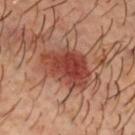No biopsy was performed on this lesion — it was imaged during a full skin examination and was not determined to be concerning.
Captured under cross-polarized illumination.
The lesion's longest dimension is about 6 mm.
The patient is a male in their 50s.
The lesion is located on the head or neck.
A 15 mm crop from a total-body photograph taken for skin-cancer surveillance.